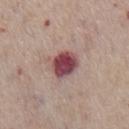The lesion was tiled from a total-body skin photograph and was not biopsied. This is a white-light tile. The patient is a male in their mid- to late 70s. From the chest. A 15 mm crop from a total-body photograph taken for skin-cancer surveillance.